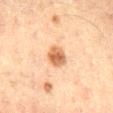Recorded during total-body skin imaging; not selected for excision or biopsy. A 15 mm close-up extracted from a 3D total-body photography capture. The lesion is on the lower back. About 2.5 mm across. A male patient, about 50 years old. The total-body-photography lesion software estimated an area of roughly 5.5 mm², a shape eccentricity near 0.4, and two-axis asymmetry of about 0.15. The analysis additionally found a border-irregularity index near 1.5/10, internal color variation of about 3.5 on a 0–10 scale, and radial color variation of about 1.